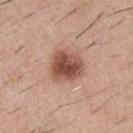  biopsy_status: not biopsied; imaged during a skin examination
  site: front of the torso
  image:
    source: total-body photography crop
    field_of_view_mm: 15
  patient:
    sex: male
    age_approx: 40